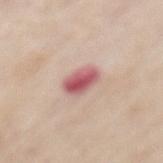This lesion was catalogued during total-body skin photography and was not selected for biopsy. The tile uses white-light illumination. This image is a 15 mm lesion crop taken from a total-body photograph. An algorithmic analysis of the crop reported an area of roughly 6.5 mm² and a symmetry-axis asymmetry near 0.15. And it measured a border-irregularity index near 1/10, internal color variation of about 6 on a 0–10 scale, and peripheral color asymmetry of about 2. And it measured a classifier nevus-likeness of about 0/100 and a detector confidence of about 100 out of 100 that the crop contains a lesion. A female subject aged around 65. The lesion's longest dimension is about 3 mm. From the mid back.